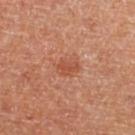Findings:
- biopsy status — catalogued during a skin exam; not biopsied
- lighting — cross-polarized illumination
- patient — female
- image — ~15 mm crop, total-body skin-cancer survey
- anatomic site — the left lower leg
- diameter — ≈3 mm